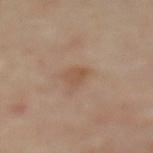Q: Was this lesion biopsied?
A: no biopsy performed (imaged during a skin exam)
Q: What are the patient's age and sex?
A: female, aged 58 to 62
Q: What is the anatomic site?
A: the back
Q: What lighting was used for the tile?
A: cross-polarized illumination
Q: What is the imaging modality?
A: total-body-photography crop, ~15 mm field of view